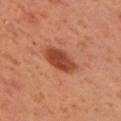Image and clinical context:
A roughly 15 mm field-of-view crop from a total-body skin photograph. The tile uses cross-polarized illumination. On the right upper arm. Automated tile analysis of the lesion measured an area of roughly 9.5 mm². The analysis additionally found a mean CIELAB color near L≈46 a*≈29 b*≈35, roughly 13 lightness units darker than nearby skin, and a normalized border contrast of about 9.5. The software also gave a border-irregularity rating of about 1.5/10, internal color variation of about 3 on a 0–10 scale, and a peripheral color-asymmetry measure near 1. The patient is a female roughly 40 years of age.